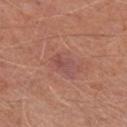The lesion was photographed on a routine skin check and not biopsied; there is no pathology result.
This is a white-light tile.
A lesion tile, about 15 mm wide, cut from a 3D total-body photograph.
A male subject, aged 63–67.
On the right lower leg.
Longest diameter approximately 2.5 mm.
The total-body-photography lesion software estimated an average lesion color of about L≈49 a*≈25 b*≈24 (CIELAB), about 6 CIELAB-L* units darker than the surrounding skin, and a normalized lesion–skin contrast near 5.